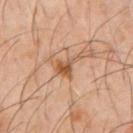image source = total-body-photography crop, ~15 mm field of view
site = the abdomen
subject = male, approximately 60 years of age